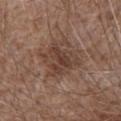anatomic site: the mid back | lighting: white-light illumination | patient: male, aged approximately 60 | lesion size: ≈4.5 mm | TBP lesion metrics: a lesion area of about 11 mm², an outline eccentricity of about 0.35 (0 = round, 1 = elongated), and a symmetry-axis asymmetry near 0.4; a mean CIELAB color near L≈40 a*≈18 b*≈25, a lesion–skin lightness drop of about 8, and a normalized lesion–skin contrast near 7; a border-irregularity index near 5/10 and a within-lesion color-variation index near 4.5/10 | imaging modality: ~15 mm crop, total-body skin-cancer survey.